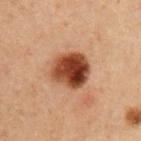- biopsy status · catalogued during a skin exam; not biopsied